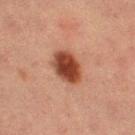biopsy status = no biopsy performed (imaged during a skin exam)
subject = female, aged approximately 55
imaging modality = 15 mm crop, total-body photography
lighting = cross-polarized illumination
site = the leg